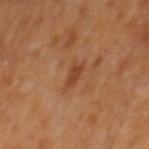Clinical impression: Imaged during a routine full-body skin examination; the lesion was not biopsied and no histopathology is available. Background: The patient is a male aged approximately 65. A 15 mm close-up tile from a total-body photography series done for melanoma screening. Measured at roughly 2.5 mm in maximum diameter. From the mid back.